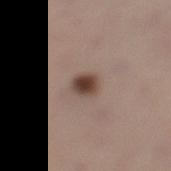Imaged during a routine full-body skin examination; the lesion was not biopsied and no histopathology is available. The lesion's longest dimension is about 3 mm. A male patient approximately 60 years of age. A 15 mm close-up tile from a total-body photography series done for melanoma screening. The tile uses white-light illumination. Located on the leg.